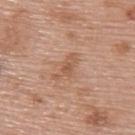Q: Was a biopsy performed?
A: imaged on a skin check; not biopsied
Q: How was this image acquired?
A: ~15 mm tile from a whole-body skin photo
Q: What lighting was used for the tile?
A: white-light illumination
Q: Where on the body is the lesion?
A: the back
Q: Automated lesion metrics?
A: an area of roughly 4.5 mm², an outline eccentricity of about 0.9 (0 = round, 1 = elongated), and a symmetry-axis asymmetry near 0.45; an average lesion color of about L≈56 a*≈21 b*≈32 (CIELAB), roughly 8 lightness units darker than nearby skin, and a normalized lesion–skin contrast near 5.5; lesion-presence confidence of about 100/100
Q: What is the lesion's diameter?
A: ≈4 mm
Q: What are the patient's age and sex?
A: female, in their 60s